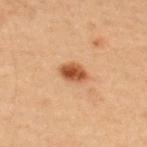Q: Patient demographics?
A: female, aged approximately 30
Q: Where on the body is the lesion?
A: the back
Q: What did automated image analysis measure?
A: an average lesion color of about L≈43 a*≈21 b*≈32 (CIELAB) and a normalized border contrast of about 10
Q: What is the imaging modality?
A: total-body-photography crop, ~15 mm field of view
Q: How large is the lesion?
A: about 3 mm
Q: What lighting was used for the tile?
A: cross-polarized illumination